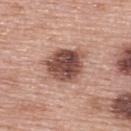{"biopsy_status": "not biopsied; imaged during a skin examination", "site": "upper back", "patient": {"sex": "female", "age_approx": 60}, "image": {"source": "total-body photography crop", "field_of_view_mm": 15}, "lesion_size": {"long_diameter_mm_approx": 5.0}, "automated_metrics": {"area_mm2_approx": 15.0, "eccentricity": 0.5, "shape_asymmetry": 0.2, "border_irregularity_0_10": 2.0, "color_variation_0_10": 6.0, "peripheral_color_asymmetry": 2.0, "nevus_likeness_0_100": 45}}